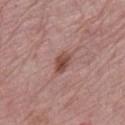<lesion>
  <biopsy_status>not biopsied; imaged during a skin examination</biopsy_status>
  <patient>
    <sex>female</sex>
    <age_approx>65</age_approx>
  </patient>
  <site>leg</site>
  <lighting>white-light</lighting>
  <lesion_size>
    <long_diameter_mm_approx>3.0</long_diameter_mm_approx>
  </lesion_size>
  <image>
    <source>total-body photography crop</source>
    <field_of_view_mm>15</field_of_view_mm>
  </image>
</lesion>